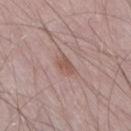Q: Was this lesion biopsied?
A: imaged on a skin check; not biopsied